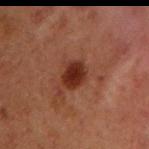Imaged during a routine full-body skin examination; the lesion was not biopsied and no histopathology is available. The lesion is on the left upper arm. The recorded lesion diameter is about 3 mm. This is a cross-polarized tile. A close-up tile cropped from a whole-body skin photograph, about 15 mm across. The subject is a male aged approximately 60.